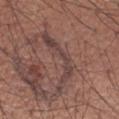The total-body-photography lesion software estimated a lesion area of about 9.5 mm², an eccentricity of roughly 0.95, and two-axis asymmetry of about 0.8. It also reported a lesion color around L≈42 a*≈18 b*≈20 in CIELAB, a lesion–skin lightness drop of about 8, and a normalized border contrast of about 7.5. And it measured border irregularity of about 10 on a 0–10 scale, internal color variation of about 1.5 on a 0–10 scale, and peripheral color asymmetry of about 0.
A lesion tile, about 15 mm wide, cut from a 3D total-body photograph.
Imaged with white-light lighting.
A male patient, aged 48 to 52.
Longest diameter approximately 8 mm.
The lesion is located on the right upper arm.
The biopsy diagnosis was a compound melanocytic nevus.